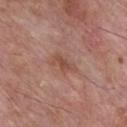lighting = white-light
location = the chest
patient = male, aged 58 to 62
image source = total-body-photography crop, ~15 mm field of view